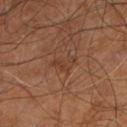biopsy status: total-body-photography surveillance lesion; no biopsy | illumination: cross-polarized | image source: 15 mm crop, total-body photography | lesion diameter: ≈3 mm | patient: male, aged approximately 60 | site: the right leg.